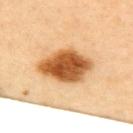Assessment: Recorded during total-body skin imaging; not selected for excision or biopsy. Context: From the upper back. Approximately 6.5 mm at its widest. Automated image analysis of the tile measured a lesion color around L≈60 a*≈25 b*≈45 in CIELAB, a lesion–skin lightness drop of about 21, and a lesion-to-skin contrast of about 12.5 (normalized; higher = more distinct). The software also gave a border-irregularity index near 1.5/10, a color-variation rating of about 6.5/10, and peripheral color asymmetry of about 2. A close-up tile cropped from a whole-body skin photograph, about 15 mm across. A female subject aged approximately 45. The tile uses cross-polarized illumination.